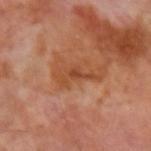{
  "biopsy_status": "not biopsied; imaged during a skin examination",
  "image": {
    "source": "total-body photography crop",
    "field_of_view_mm": 15
  },
  "site": "arm",
  "patient": {
    "sex": "male",
    "age_approx": 70
  }
}